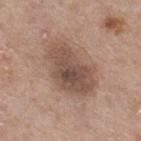| feature | finding |
|---|---|
| workup | catalogued during a skin exam; not biopsied |
| imaging modality | 15 mm crop, total-body photography |
| automated metrics | a border-irregularity rating of about 2.5/10, internal color variation of about 5 on a 0–10 scale, and peripheral color asymmetry of about 1.5 |
| patient | male, aged 53–57 |
| lesion diameter | ~7 mm (longest diameter) |
| lighting | white-light |